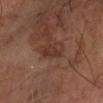Part of a total-body skin-imaging series; this lesion was reviewed on a skin check and was not flagged for biopsy.